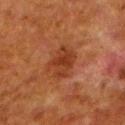Q: Lesion size?
A: ≈4 mm
Q: Lesion location?
A: the left lower leg
Q: How was this image acquired?
A: 15 mm crop, total-body photography
Q: What are the patient's age and sex?
A: male, approximately 80 years of age
Q: What did automated image analysis measure?
A: a lesion color around L≈30 a*≈23 b*≈29 in CIELAB, about 7 CIELAB-L* units darker than the surrounding skin, and a lesion-to-skin contrast of about 7 (normalized; higher = more distinct)